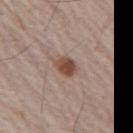<case>
  <biopsy_status>not biopsied; imaged during a skin examination</biopsy_status>
  <image>
    <source>total-body photography crop</source>
    <field_of_view_mm>15</field_of_view_mm>
  </image>
  <patient>
    <sex>male</sex>
    <age_approx>65</age_approx>
  </patient>
  <site>arm</site>
</case>